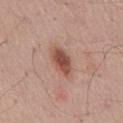subject — male, aged 53 to 57 | body site — the abdomen | image-analysis metrics — a lesion area of about 7.5 mm², a shape eccentricity near 0.85, and two-axis asymmetry of about 0.15; a border-irregularity index near 1.5/10 and a peripheral color-asymmetry measure near 1 | acquisition — ~15 mm tile from a whole-body skin photo | size — ≈4 mm | illumination — white-light illumination.